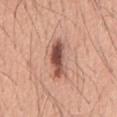Recorded during total-body skin imaging; not selected for excision or biopsy.
Located on the back.
The patient is a male approximately 60 years of age.
The lesion-visualizer software estimated an area of roughly 9 mm² and an eccentricity of roughly 0.95. The analysis additionally found an automated nevus-likeness rating near 95 out of 100.
This image is a 15 mm lesion crop taken from a total-body photograph.
The lesion's longest dimension is about 5.5 mm.
This is a white-light tile.